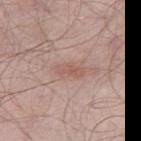biopsy status — imaged on a skin check; not biopsied
image — total-body-photography crop, ~15 mm field of view
size — ~3.5 mm (longest diameter)
location — the left thigh
subject — male, in their mid-50s
tile lighting — white-light